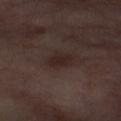Q: How was the tile lit?
A: cross-polarized illumination
Q: What kind of image is this?
A: ~15 mm tile from a whole-body skin photo
Q: What is the anatomic site?
A: the right thigh
Q: What are the patient's age and sex?
A: female, in their mid- to late 50s